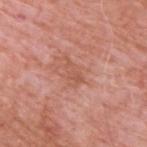biopsy status=no biopsy performed (imaged during a skin exam)
anatomic site=the upper back
subject=male, aged around 60
illumination=white-light illumination
acquisition=total-body-photography crop, ~15 mm field of view
lesion size=about 3.5 mm